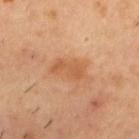Notes:
• notes — no biopsy performed (imaged during a skin exam)
• lesion diameter — about 4 mm
• image source — 15 mm crop, total-body photography
• location — the upper back
• patient — male, roughly 55 years of age
• tile lighting — cross-polarized illumination